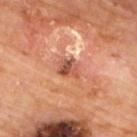Impression: Imaged during a routine full-body skin examination; the lesion was not biopsied and no histopathology is available. Context: Captured under cross-polarized illumination. The lesion is located on the back. The subject is a male aged 58 to 62. A 15 mm close-up tile from a total-body photography series done for melanoma screening. Approximately 2.5 mm at its widest.